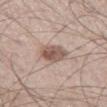The lesion was photographed on a routine skin check and not biopsied; there is no pathology result. The lesion is located on the left thigh. Approximately 3.5 mm at its widest. Captured under white-light illumination. A roughly 15 mm field-of-view crop from a total-body skin photograph. The patient is a male aged around 60.